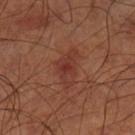| feature | finding |
|---|---|
| biopsy status | total-body-photography surveillance lesion; no biopsy |
| TBP lesion metrics | a shape eccentricity near 0.8 and two-axis asymmetry of about 0.4; an average lesion color of about L≈34 a*≈24 b*≈26 (CIELAB), roughly 6 lightness units darker than nearby skin, and a lesion-to-skin contrast of about 6 (normalized; higher = more distinct); a detector confidence of about 100 out of 100 that the crop contains a lesion |
| imaging modality | total-body-photography crop, ~15 mm field of view |
| lesion diameter | about 4.5 mm |
| patient | male, in their 70s |
| location | the left lower leg |
| lighting | cross-polarized illumination |